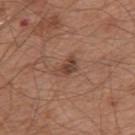Recorded during total-body skin imaging; not selected for excision or biopsy.
On the back.
This image is a 15 mm lesion crop taken from a total-body photograph.
The subject is a male aged 63–67.
The total-body-photography lesion software estimated an automated nevus-likeness rating near 45 out of 100 and a lesion-detection confidence of about 100/100.